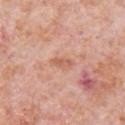Clinical impression:
This lesion was catalogued during total-body skin photography and was not selected for biopsy.
Context:
Cropped from a total-body skin-imaging series; the visible field is about 15 mm. A male subject approximately 80 years of age. From the chest.